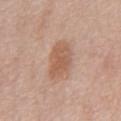Clinical impression:
The lesion was tiled from a total-body skin photograph and was not biopsied.
Acquisition and patient details:
A male subject aged 73 to 77. The tile uses white-light illumination. Approximately 4 mm at its widest. A 15 mm close-up extracted from a 3D total-body photography capture. Located on the abdomen.